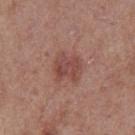Imaged during a routine full-body skin examination; the lesion was not biopsied and no histopathology is available.
A female patient, roughly 40 years of age.
This is a white-light tile.
The lesion is located on the right thigh.
The recorded lesion diameter is about 3.5 mm.
An algorithmic analysis of the crop reported a lesion color around L≈46 a*≈23 b*≈24 in CIELAB, a lesion–skin lightness drop of about 9, and a lesion-to-skin contrast of about 6.5 (normalized; higher = more distinct). And it measured a within-lesion color-variation index near 3.5/10 and radial color variation of about 1.
A 15 mm close-up extracted from a 3D total-body photography capture.